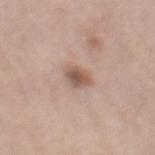notes: total-body-photography surveillance lesion; no biopsy.